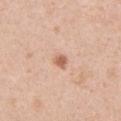Impression: No biopsy was performed on this lesion — it was imaged during a full skin examination and was not determined to be concerning. Context: Cropped from a whole-body photographic skin survey; the tile spans about 15 mm. The recorded lesion diameter is about 3 mm. The lesion is on the left upper arm. A female subject, aged 43 to 47. This is a white-light tile. An algorithmic analysis of the crop reported a lesion area of about 5.5 mm², an eccentricity of roughly 0.65, and two-axis asymmetry of about 0.1. The software also gave an average lesion color of about L≈67 a*≈20 b*≈31 (CIELAB), a lesion–skin lightness drop of about 8, and a normalized lesion–skin contrast near 5.5. And it measured a nevus-likeness score of about 70/100 and a detector confidence of about 100 out of 100 that the crop contains a lesion.